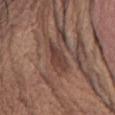This lesion was catalogued during total-body skin photography and was not selected for biopsy. Cropped from a total-body skin-imaging series; the visible field is about 15 mm. A male patient, aged 68–72. On the abdomen. The tile uses white-light illumination. The recorded lesion diameter is about 3.5 mm.